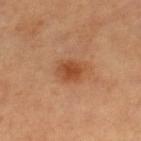The lesion is on the leg. Approximately 3.5 mm at its widest. A female subject aged approximately 60. This image is a 15 mm lesion crop taken from a total-body photograph. The tile uses cross-polarized illumination. An algorithmic analysis of the crop reported a classifier nevus-likeness of about 90/100 and lesion-presence confidence of about 100/100.